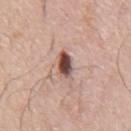* workup — catalogued during a skin exam; not biopsied
* acquisition — ~15 mm tile from a whole-body skin photo
* lesion size — ~3.5 mm (longest diameter)
* image-analysis metrics — a footprint of about 5 mm², an eccentricity of roughly 0.85, and two-axis asymmetry of about 0.2; an average lesion color of about L≈49 a*≈21 b*≈23 (CIELAB) and a lesion-to-skin contrast of about 13 (normalized; higher = more distinct); a border-irregularity index near 2/10, internal color variation of about 7.5 on a 0–10 scale, and a peripheral color-asymmetry measure near 2.5
* illumination — white-light illumination
* patient — male, aged around 75
* body site — the abdomen